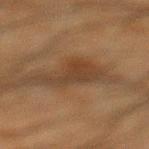Impression: The lesion was photographed on a routine skin check and not biopsied; there is no pathology result. Acquisition and patient details: A 15 mm close-up extracted from a 3D total-body photography capture. A male patient, about 35 years old. Located on the left lower leg. Automated image analysis of the tile measured a footprint of about 11 mm², a shape eccentricity near 0.75, and a symmetry-axis asymmetry near 0.5. The analysis additionally found an automated nevus-likeness rating near 0 out of 100 and lesion-presence confidence of about 95/100. Captured under cross-polarized illumination.